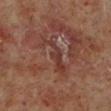Assessment: The lesion was tiled from a total-body skin photograph and was not biopsied. Image and clinical context: The lesion is on the left lower leg. Cropped from a whole-body photographic skin survey; the tile spans about 15 mm. A male patient approximately 60 years of age. Approximately 5.5 mm at its widest. This is a cross-polarized tile.